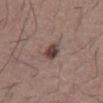| key | value |
|---|---|
| workup | no biopsy performed (imaged during a skin exam) |
| subject | male, aged around 40 |
| image | total-body-photography crop, ~15 mm field of view |
| location | the front of the torso |
| tile lighting | white-light illumination |
| size | ≈2.5 mm |